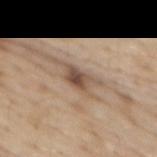Q: Was a biopsy performed?
A: total-body-photography surveillance lesion; no biopsy
Q: Lesion location?
A: the abdomen
Q: Lesion size?
A: ~4 mm (longest diameter)
Q: What kind of image is this?
A: ~15 mm tile from a whole-body skin photo
Q: What are the patient's age and sex?
A: male, about 70 years old
Q: How was the tile lit?
A: white-light